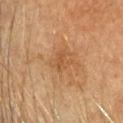<tbp_lesion>
<biopsy_status>not biopsied; imaged during a skin examination</biopsy_status>
<automated_metrics>
  <cielab_L>46</cielab_L>
  <cielab_a>19</cielab_a>
  <cielab_b>34</cielab_b>
  <vs_skin_darker_L>6.0</vs_skin_darker_L>
</automated_metrics>
<lesion_size>
  <long_diameter_mm_approx>2.5</long_diameter_mm_approx>
</lesion_size>
<image>
  <source>total-body photography crop</source>
  <field_of_view_mm>15</field_of_view_mm>
</image>
<site>head or neck</site>
<patient>
  <sex>female</sex>
  <age_approx>55</age_approx>
</patient>
<lighting>cross-polarized</lighting>
</tbp_lesion>